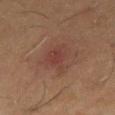follow-up — imaged on a skin check; not biopsied | image-analysis metrics — a mean CIELAB color near L≈38 a*≈21 b*≈23, a lesion–skin lightness drop of about 6, and a lesion-to-skin contrast of about 5.5 (normalized; higher = more distinct) | tile lighting — cross-polarized | image — total-body-photography crop, ~15 mm field of view | patient — male, aged 58 to 62 | location — the right thigh.